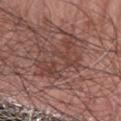The lesion-visualizer software estimated an area of roughly 14 mm², an eccentricity of roughly 0.85, and a shape-asymmetry score of about 0.55 (0 = symmetric).
The subject is a male aged 58–62.
A roughly 15 mm field-of-view crop from a total-body skin photograph.
Located on the right forearm.